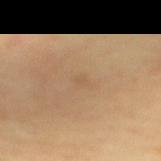Impression: No biopsy was performed on this lesion — it was imaged during a full skin examination and was not determined to be concerning. Context: Imaged with cross-polarized lighting. The subject is a male in their mid-50s. A 15 mm close-up extracted from a 3D total-body photography capture. From the mid back. An algorithmic analysis of the crop reported a symmetry-axis asymmetry near 0.3. The analysis additionally found a mean CIELAB color near L≈56 a*≈16 b*≈34, roughly 4 lightness units darker than nearby skin, and a normalized border contrast of about 3. The software also gave a nevus-likeness score of about 0/100 and lesion-presence confidence of about 100/100. About 1.5 mm across.